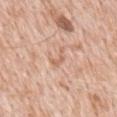Clinical impression:
No biopsy was performed on this lesion — it was imaged during a full skin examination and was not determined to be concerning.
Image and clinical context:
This is a white-light tile. Located on the mid back. Longest diameter approximately 2.5 mm. A close-up tile cropped from a whole-body skin photograph, about 15 mm across. A male patient, approximately 50 years of age.